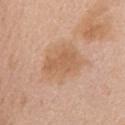This lesion was catalogued during total-body skin photography and was not selected for biopsy. The lesion-visualizer software estimated a footprint of about 12 mm², an eccentricity of roughly 0.7, and a shape-asymmetry score of about 0.25 (0 = symmetric). It also reported a lesion color around L≈60 a*≈21 b*≈34 in CIELAB, roughly 8 lightness units darker than nearby skin, and a lesion-to-skin contrast of about 6 (normalized; higher = more distinct). And it measured a border-irregularity rating of about 3/10, internal color variation of about 2 on a 0–10 scale, and radial color variation of about 0.5. And it measured a detector confidence of about 100 out of 100 that the crop contains a lesion. Measured at roughly 4.5 mm in maximum diameter. The subject is a female aged approximately 65. This is a white-light tile. The lesion is on the mid back. Cropped from a whole-body photographic skin survey; the tile spans about 15 mm.